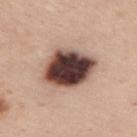Captured during whole-body skin photography for melanoma surveillance; the lesion was not biopsied. From the upper back. The patient is a male aged 43 to 47. Measured at roughly 6.5 mm in maximum diameter. The tile uses white-light illumination. Cropped from a total-body skin-imaging series; the visible field is about 15 mm.